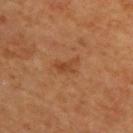Notes:
• workup · no biopsy performed (imaged during a skin exam)
• lesion size · ~3 mm (longest diameter)
• image-analysis metrics · a footprint of about 4 mm², an eccentricity of roughly 0.8, and a shape-asymmetry score of about 0.5 (0 = symmetric); about 7 CIELAB-L* units darker than the surrounding skin and a normalized border contrast of about 5.5; internal color variation of about 2 on a 0–10 scale and a peripheral color-asymmetry measure near 0.5; an automated nevus-likeness rating near 5 out of 100 and a lesion-detection confidence of about 100/100
• subject · male, aged around 65
• acquisition · total-body-photography crop, ~15 mm field of view
• anatomic site · the upper back